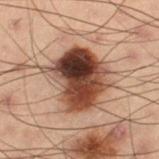Impression:
This lesion was catalogued during total-body skin photography and was not selected for biopsy.
Background:
Imaged with cross-polarized lighting. A male patient in their mid-50s. Located on the left thigh. Approximately 6 mm at its widest. A 15 mm close-up tile from a total-body photography series done for melanoma screening.